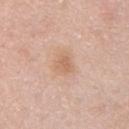The lesion was tiled from a total-body skin photograph and was not biopsied.